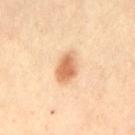Imaged with cross-polarized lighting. A female subject, aged approximately 40. The lesion is located on the lower back. Longest diameter approximately 4 mm. A lesion tile, about 15 mm wide, cut from a 3D total-body photograph.